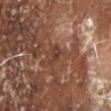Part of a total-body skin-imaging series; this lesion was reviewed on a skin check and was not flagged for biopsy. From the head or neck. A 15 mm close-up extracted from a 3D total-body photography capture. A male subject, approximately 80 years of age.